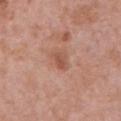Case summary:
* biopsy status · total-body-photography surveillance lesion; no biopsy
* size · ≈2.5 mm
* patient · female, approximately 40 years of age
* body site · the front of the torso
* imaging modality · ~15 mm crop, total-body skin-cancer survey